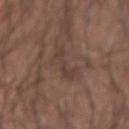workup = imaged on a skin check; not biopsied | anatomic site = the left forearm | patient = male, aged around 35 | imaging modality = 15 mm crop, total-body photography.